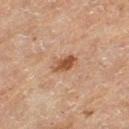Q: Is there a histopathology result?
A: catalogued during a skin exam; not biopsied